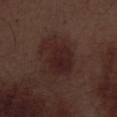The lesion was tiled from a total-body skin photograph and was not biopsied. The lesion's longest dimension is about 5 mm. From the leg. This is a white-light tile. A 15 mm close-up extracted from a 3D total-body photography capture. The subject is a male aged around 70.